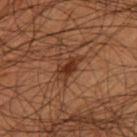Q: Was this lesion biopsied?
A: total-body-photography surveillance lesion; no biopsy
Q: Patient demographics?
A: male, aged 53–57
Q: Automated lesion metrics?
A: an eccentricity of roughly 0.85 and a shape-asymmetry score of about 0.3 (0 = symmetric); a lesion-detection confidence of about 100/100
Q: What is the imaging modality?
A: ~15 mm tile from a whole-body skin photo
Q: Where on the body is the lesion?
A: the leg
Q: How was the tile lit?
A: cross-polarized